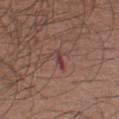The lesion was tiled from a total-body skin photograph and was not biopsied.
The lesion's longest dimension is about 2.5 mm.
Cropped from a total-body skin-imaging series; the visible field is about 15 mm.
A male patient, aged 18 to 22.
On the leg.
The lesion-visualizer software estimated an area of roughly 2.5 mm², a shape eccentricity near 0.9, and a shape-asymmetry score of about 0.25 (0 = symmetric). The software also gave a border-irregularity index near 3/10, a within-lesion color-variation index near 0/10, and peripheral color asymmetry of about 0. And it measured a nevus-likeness score of about 0/100 and lesion-presence confidence of about 90/100.